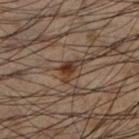Case summary:
• diameter — ≈3.5 mm
• anatomic site — the leg
• acquisition — ~15 mm tile from a whole-body skin photo
• image-analysis metrics — a border-irregularity rating of about 5.5/10 and radial color variation of about 1; lesion-presence confidence of about 95/100
• illumination — cross-polarized illumination
• patient — male, roughly 50 years of age